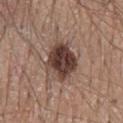Q: Is there a histopathology result?
A: total-body-photography surveillance lesion; no biopsy
Q: Where on the body is the lesion?
A: the back
Q: What are the patient's age and sex?
A: male, approximately 65 years of age
Q: What kind of image is this?
A: 15 mm crop, total-body photography
Q: How large is the lesion?
A: ~5 mm (longest diameter)
Q: How was the tile lit?
A: white-light